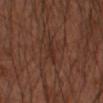Impression:
The lesion was photographed on a routine skin check and not biopsied; there is no pathology result.
Background:
A roughly 15 mm field-of-view crop from a total-body skin photograph. The tile uses cross-polarized illumination. An algorithmic analysis of the crop reported a lesion color around L≈26 a*≈17 b*≈22 in CIELAB, about 5 CIELAB-L* units darker than the surrounding skin, and a normalized border contrast of about 5.5. It also reported an automated nevus-likeness rating near 0 out of 100 and lesion-presence confidence of about 55/100. On the left forearm. A male subject, aged 43–47.